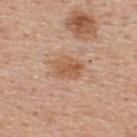Q: Is there a histopathology result?
A: no biopsy performed (imaged during a skin exam)
Q: Patient demographics?
A: male, roughly 55 years of age
Q: Where on the body is the lesion?
A: the upper back
Q: What did automated image analysis measure?
A: a footprint of about 7 mm², an eccentricity of roughly 0.7, and a symmetry-axis asymmetry near 0.2; a lesion color around L≈57 a*≈22 b*≈34 in CIELAB, roughly 9 lightness units darker than nearby skin, and a normalized lesion–skin contrast near 7; a border-irregularity rating of about 2/10, a within-lesion color-variation index near 3.5/10, and a peripheral color-asymmetry measure near 1.5; a classifier nevus-likeness of about 65/100 and a detector confidence of about 100 out of 100 that the crop contains a lesion
Q: How was the tile lit?
A: white-light illumination
Q: Lesion size?
A: ~3.5 mm (longest diameter)
Q: What is the imaging modality?
A: 15 mm crop, total-body photography